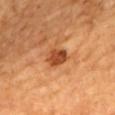Part of a total-body skin-imaging series; this lesion was reviewed on a skin check and was not flagged for biopsy. The recorded lesion diameter is about 3 mm. The lesion is located on the mid back. A male patient aged approximately 65. This image is a 15 mm lesion crop taken from a total-body photograph.